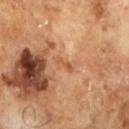• follow-up: total-body-photography surveillance lesion; no biopsy
• tile lighting: cross-polarized
• subject: male, approximately 65 years of age
• diameter: ~2.5 mm (longest diameter)
• image: ~15 mm crop, total-body skin-cancer survey